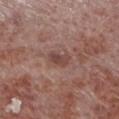Findings:
* subject · male, aged around 55
* lesion size · ≈2.5 mm
* imaging modality · 15 mm crop, total-body photography
* illumination · white-light
* anatomic site · the left lower leg